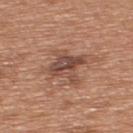anatomic site: the upper back | image source: ~15 mm tile from a whole-body skin photo | size: ~4.5 mm (longest diameter) | lighting: white-light | patient: male, in their mid- to late 60s.